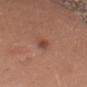Notes:
• image source — total-body-photography crop, ~15 mm field of view
• image-analysis metrics — an eccentricity of roughly 0.9 and a symmetry-axis asymmetry near 0.25; a mean CIELAB color near L≈45 a*≈23 b*≈28 and a normalized lesion–skin contrast near 7; border irregularity of about 2.5 on a 0–10 scale and peripheral color asymmetry of about 0.5
• illumination — white-light illumination
• lesion size — ≈3 mm
• location — the upper back
• subject — male, in their 70s